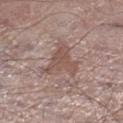biopsy status: total-body-photography surveillance lesion; no biopsy
imaging modality: 15 mm crop, total-body photography
site: the right lower leg
automated lesion analysis: a shape-asymmetry score of about 0.65 (0 = symmetric); a mean CIELAB color near L≈52 a*≈18 b*≈22 and a lesion-to-skin contrast of about 6 (normalized; higher = more distinct)
subject: male, in their mid- to late 60s
lighting: white-light illumination
diameter: ~4.5 mm (longest diameter)